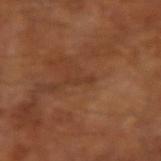biopsy status: no biopsy performed (imaged during a skin exam) | imaging modality: ~15 mm tile from a whole-body skin photo | subject: male, aged approximately 65.